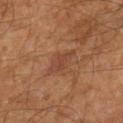follow-up: imaged on a skin check; not biopsied
site: the right lower leg
lighting: cross-polarized illumination
acquisition: ~15 mm crop, total-body skin-cancer survey
subject: male, about 60 years old